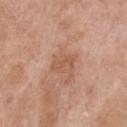biopsy_status: not biopsied; imaged during a skin examination
patient:
  sex: female
  age_approx: 60
site: chest
image:
  source: total-body photography crop
  field_of_view_mm: 15
lesion_size:
  long_diameter_mm_approx: 3.5
lighting: white-light